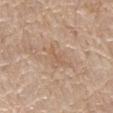biopsy status = no biopsy performed (imaged during a skin exam)
location = the left forearm
image-analysis metrics = a footprint of about 2.5 mm²; an average lesion color of about L≈58 a*≈18 b*≈32 (CIELAB) and a lesion–skin lightness drop of about 6; a border-irregularity index near 6/10 and a color-variation rating of about 0/10; an automated nevus-likeness rating near 0 out of 100 and a lesion-detection confidence of about 100/100
patient = female, approximately 65 years of age
acquisition = total-body-photography crop, ~15 mm field of view
lesion size = ~3 mm (longest diameter)